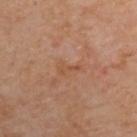<tbp_lesion>
  <biopsy_status>not biopsied; imaged during a skin examination</biopsy_status>
  <site>back</site>
  <patient>
    <sex>female</sex>
    <age_approx>60</age_approx>
  </patient>
  <lesion_size>
    <long_diameter_mm_approx>3.0</long_diameter_mm_approx>
  </lesion_size>
  <image>
    <source>total-body photography crop</source>
    <field_of_view_mm>15</field_of_view_mm>
  </image>
</tbp_lesion>